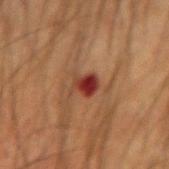follow-up: catalogued during a skin exam; not biopsied
site: the arm
size: about 3 mm
TBP lesion metrics: a lesion area of about 5.5 mm² and a shape-asymmetry score of about 0.3 (0 = symmetric); a color-variation rating of about 6.5/10 and peripheral color asymmetry of about 2; a nevus-likeness score of about 0/100 and a detector confidence of about 100 out of 100 that the crop contains a lesion
image source: ~15 mm tile from a whole-body skin photo
patient: male, roughly 55 years of age
tile lighting: cross-polarized illumination